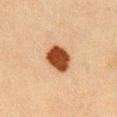<case>
  <biopsy_status>not biopsied; imaged during a skin examination</biopsy_status>
  <patient>
    <sex>female</sex>
    <age_approx>20</age_approx>
  </patient>
  <site>chest</site>
  <automated_metrics>
    <area_mm2_approx>10.0</area_mm2_approx>
    <eccentricity>0.6</eccentricity>
    <vs_skin_contrast_norm>14.0</vs_skin_contrast_norm>
    <peripheral_color_asymmetry>1.0</peripheral_color_asymmetry>
    <nevus_likeness_0_100>100</nevus_likeness_0_100>
  </automated_metrics>
  <image>
    <source>total-body photography crop</source>
    <field_of_view_mm>15</field_of_view_mm>
  </image>
  <lesion_size>
    <long_diameter_mm_approx>3.5</long_diameter_mm_approx>
  </lesion_size>
  <lighting>cross-polarized</lighting>
</case>